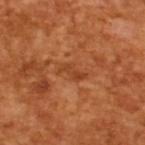workup — total-body-photography surveillance lesion; no biopsy | image source — ~15 mm crop, total-body skin-cancer survey | illumination — cross-polarized illumination | lesion size — ~3 mm (longest diameter) | automated lesion analysis — a lesion area of about 2.5 mm², a shape eccentricity near 0.95, and a shape-asymmetry score of about 0.5 (0 = symmetric); an average lesion color of about L≈41 a*≈28 b*≈39 (CIELAB) and roughly 8 lightness units darker than nearby skin; a border-irregularity index near 5.5/10, a color-variation rating of about 0/10, and peripheral color asymmetry of about 0; a nevus-likeness score of about 0/100 and a detector confidence of about 95 out of 100 that the crop contains a lesion | subject — male, roughly 65 years of age.